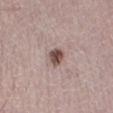Assessment:
The lesion was tiled from a total-body skin photograph and was not biopsied.
Image and clinical context:
The patient is a female in their 50s. The lesion-visualizer software estimated an average lesion color of about L≈49 a*≈18 b*≈21 (CIELAB), roughly 15 lightness units darker than nearby skin, and a normalized border contrast of about 10. It also reported internal color variation of about 4 on a 0–10 scale and radial color variation of about 1. Captured under white-light illumination. This image is a 15 mm lesion crop taken from a total-body photograph. Located on the right lower leg.